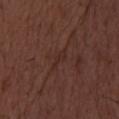Q: Is there a histopathology result?
A: no biopsy performed (imaged during a skin exam)
Q: What is the imaging modality?
A: total-body-photography crop, ~15 mm field of view
Q: What are the patient's age and sex?
A: male, in their 50s